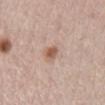Part of a total-body skin-imaging series; this lesion was reviewed on a skin check and was not flagged for biopsy.
A female patient, in their mid- to late 60s.
On the abdomen.
Automated image analysis of the tile measured a footprint of about 8 mm² and two-axis asymmetry of about 0.25.
A 15 mm close-up extracted from a 3D total-body photography capture.